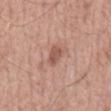follow-up=no biopsy performed (imaged during a skin exam)
image source=~15 mm crop, total-body skin-cancer survey
subject=male, aged approximately 55
illumination=white-light illumination
lesion size=~3 mm (longest diameter)
location=the back
TBP lesion metrics=a classifier nevus-likeness of about 0/100 and a lesion-detection confidence of about 100/100